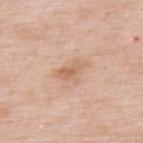Clinical impression: The lesion was tiled from a total-body skin photograph and was not biopsied. Background: The lesion is located on the upper back. The lesion-visualizer software estimated an eccentricity of roughly 0.8 and two-axis asymmetry of about 0.45. And it measured an average lesion color of about L≈64 a*≈20 b*≈33 (CIELAB) and about 8 CIELAB-L* units darker than the surrounding skin. The analysis additionally found a nevus-likeness score of about 5/100 and a lesion-detection confidence of about 100/100. A male patient, aged 53 to 57. This is a white-light tile. About 3.5 mm across. Cropped from a whole-body photographic skin survey; the tile spans about 15 mm.